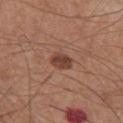Part of a total-body skin-imaging series; this lesion was reviewed on a skin check and was not flagged for biopsy. Captured under white-light illumination. A roughly 15 mm field-of-view crop from a total-body skin photograph. From the chest. About 2.5 mm across. The subject is a male in their mid- to late 60s.